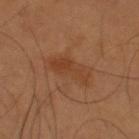Assessment: The lesion was tiled from a total-body skin photograph and was not biopsied. Image and clinical context: A male patient aged approximately 55. From the upper back. A close-up tile cropped from a whole-body skin photograph, about 15 mm across. Imaged with cross-polarized lighting. An algorithmic analysis of the crop reported a shape eccentricity near 0.9 and a shape-asymmetry score of about 0.25 (0 = symmetric). And it measured a lesion color around L≈40 a*≈21 b*≈32 in CIELAB, about 6 CIELAB-L* units darker than the surrounding skin, and a lesion-to-skin contrast of about 5.5 (normalized; higher = more distinct). The software also gave border irregularity of about 3.5 on a 0–10 scale, internal color variation of about 3.5 on a 0–10 scale, and radial color variation of about 1.5. It also reported an automated nevus-likeness rating near 0 out of 100 and lesion-presence confidence of about 100/100.